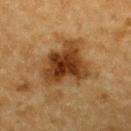workup: imaged on a skin check; not biopsied
subject: male, aged approximately 85 or older
anatomic site: the back
lighting: cross-polarized
image-analysis metrics: internal color variation of about 6 on a 0–10 scale and radial color variation of about 1.5
imaging modality: ~15 mm tile from a whole-body skin photo